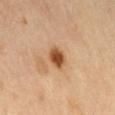automated lesion analysis: a mean CIELAB color near L≈50 a*≈23 b*≈37 and a normalized border contrast of about 11; a border-irregularity index near 1.5/10, a within-lesion color-variation index near 3.5/10, and radial color variation of about 1; an automated nevus-likeness rating near 95 out of 100 and lesion-presence confidence of about 100/100 | subject: male, roughly 70 years of age | anatomic site: the front of the torso | imaging modality: ~15 mm crop, total-body skin-cancer survey | lesion size: ≈2.5 mm.